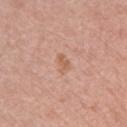The lesion was tiled from a total-body skin photograph and was not biopsied. A female patient aged approximately 60. A roughly 15 mm field-of-view crop from a total-body skin photograph. Longest diameter approximately 2.5 mm. The tile uses white-light illumination. Located on the left upper arm.